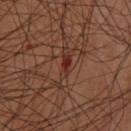Clinical impression: The lesion was tiled from a total-body skin photograph and was not biopsied. Acquisition and patient details: About 3 mm across. This image is a 15 mm lesion crop taken from a total-body photograph. A female subject roughly 45 years of age. Captured under cross-polarized illumination. The lesion is located on the upper back. The lesion-visualizer software estimated a footprint of about 3 mm² and an eccentricity of roughly 0.9. It also reported a mean CIELAB color near L≈30 a*≈24 b*≈26 and a lesion–skin lightness drop of about 8. And it measured a border-irregularity rating of about 2.5/10 and internal color variation of about 1.5 on a 0–10 scale.